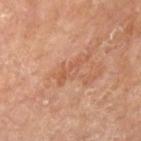Assessment: Recorded during total-body skin imaging; not selected for excision or biopsy. Image and clinical context: A male subject, approximately 85 years of age or older. A 15 mm close-up extracted from a 3D total-body photography capture. The lesion is on the left upper arm.